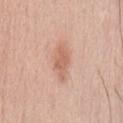Part of a total-body skin-imaging series; this lesion was reviewed on a skin check and was not flagged for biopsy. The subject is a female aged around 60. From the abdomen. A roughly 15 mm field-of-view crop from a total-body skin photograph. About 4.5 mm across. Captured under white-light illumination.